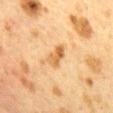Impression: Captured during whole-body skin photography for melanoma surveillance; the lesion was not biopsied. Clinical summary: The subject is a female about 40 years old. A 15 mm crop from a total-body photograph taken for skin-cancer surveillance. Captured under cross-polarized illumination. The lesion is on the mid back. About 3 mm across. An algorithmic analysis of the crop reported a mean CIELAB color near L≈54 a*≈18 b*≈39 and about 9 CIELAB-L* units darker than the surrounding skin.